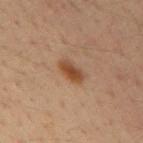follow-up: imaged on a skin check; not biopsied
image-analysis metrics: an outline eccentricity of about 0.85 (0 = round, 1 = elongated); an automated nevus-likeness rating near 100 out of 100 and a detector confidence of about 100 out of 100 that the crop contains a lesion
acquisition: ~15 mm tile from a whole-body skin photo
subject: male, aged 33–37
lighting: cross-polarized
anatomic site: the right upper arm
lesion size: about 3.5 mm